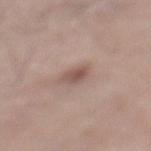This lesion was catalogued during total-body skin photography and was not selected for biopsy.
The tile uses white-light illumination.
A male patient approximately 65 years of age.
Cropped from a total-body skin-imaging series; the visible field is about 15 mm.
The lesion is located on the left lower leg.